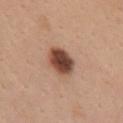workup — catalogued during a skin exam; not biopsied
illumination — white-light
lesion diameter — ~4 mm (longest diameter)
location — the chest
subject — female, aged approximately 30
automated lesion analysis — an area of roughly 9 mm², a shape eccentricity near 0.7, and a shape-asymmetry score of about 0.1 (0 = symmetric); an average lesion color of about L≈46 a*≈22 b*≈28 (CIELAB), about 18 CIELAB-L* units darker than the surrounding skin, and a normalized border contrast of about 13; a border-irregularity index near 1/10, a color-variation rating of about 5.5/10, and radial color variation of about 1.5
imaging modality — ~15 mm crop, total-body skin-cancer survey